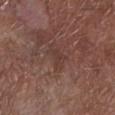Q: Was this lesion biopsied?
A: no biopsy performed (imaged during a skin exam)
Q: How was the tile lit?
A: white-light
Q: How large is the lesion?
A: ≈3 mm
Q: Automated lesion metrics?
A: an area of roughly 4 mm², an eccentricity of roughly 0.75, and two-axis asymmetry of about 0.4; a mean CIELAB color near L≈37 a*≈19 b*≈22 and roughly 5 lightness units darker than nearby skin; a border-irregularity rating of about 4/10 and radial color variation of about 0.5
Q: What are the patient's age and sex?
A: male, aged 53 to 57
Q: What kind of image is this?
A: ~15 mm tile from a whole-body skin photo
Q: Lesion location?
A: the left lower leg